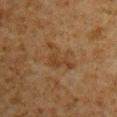Clinical impression:
This lesion was catalogued during total-body skin photography and was not selected for biopsy.
Clinical summary:
Imaged with cross-polarized lighting. From the right upper arm. A 15 mm close-up tile from a total-body photography series done for melanoma screening. Longest diameter approximately 5 mm. Automated image analysis of the tile measured a lesion area of about 7.5 mm², an outline eccentricity of about 0.8 (0 = round, 1 = elongated), and a shape-asymmetry score of about 0.6 (0 = symmetric). The software also gave a lesion color around L≈34 a*≈16 b*≈29 in CIELAB, roughly 6 lightness units darker than nearby skin, and a normalized border contrast of about 6. It also reported a border-irregularity rating of about 7.5/10, a within-lesion color-variation index near 1.5/10, and peripheral color asymmetry of about 0.5. A male subject aged 58 to 62.